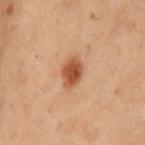Assessment: No biopsy was performed on this lesion — it was imaged during a full skin examination and was not determined to be concerning. Context: The lesion is on the front of the torso. This is a cross-polarized tile. The subject is a male aged approximately 70. The lesion's longest dimension is about 3.5 mm. Automated image analysis of the tile measured a mean CIELAB color near L≈39 a*≈20 b*≈29, roughly 11 lightness units darker than nearby skin, and a normalized border contrast of about 9.5. A close-up tile cropped from a whole-body skin photograph, about 15 mm across.